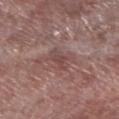Recorded during total-body skin imaging; not selected for excision or biopsy.
Captured under white-light illumination.
Located on the left lower leg.
The subject is a male aged 53 to 57.
About 3.5 mm across.
Automated image analysis of the tile measured a lesion area of about 4 mm², an outline eccentricity of about 0.9 (0 = round, 1 = elongated), and two-axis asymmetry of about 0.3.
A close-up tile cropped from a whole-body skin photograph, about 15 mm across.